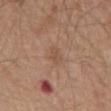Q: Is there a histopathology result?
A: no biopsy performed (imaged during a skin exam)